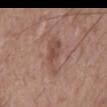{"site": "mid back", "image": {"source": "total-body photography crop", "field_of_view_mm": 15}, "patient": {"sex": "male", "age_approx": 60}, "lighting": "white-light"}